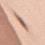Imaged during a routine full-body skin examination; the lesion was not biopsied and no histopathology is available. Cropped from a total-body skin-imaging series; the visible field is about 15 mm. This is a white-light tile. A female subject about 50 years old. Longest diameter approximately 5.5 mm. On the back. An algorithmic analysis of the crop reported an area of roughly 8.5 mm², an eccentricity of roughly 0.95, and a shape-asymmetry score of about 0.2 (0 = symmetric). And it measured border irregularity of about 3.5 on a 0–10 scale, a within-lesion color-variation index near 10/10, and radial color variation of about 3.5. The analysis additionally found an automated nevus-likeness rating near 95 out of 100 and a detector confidence of about 100 out of 100 that the crop contains a lesion.